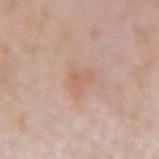{
  "biopsy_status": "not biopsied; imaged during a skin examination",
  "image": {
    "source": "total-body photography crop",
    "field_of_view_mm": 15
  },
  "lighting": "white-light",
  "site": "right forearm",
  "automated_metrics": {
    "area_mm2_approx": 2.0,
    "eccentricity": 0.85,
    "cielab_L": 61,
    "cielab_a": 20,
    "cielab_b": 29,
    "vs_skin_darker_L": 6.0,
    "vs_skin_contrast_norm": 4.5
  },
  "lesion_size": {
    "long_diameter_mm_approx": 2.5
  },
  "patient": {
    "sex": "female",
    "age_approx": 60
  }
}